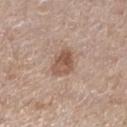Q: Was this lesion biopsied?
A: imaged on a skin check; not biopsied
Q: What kind of image is this?
A: ~15 mm crop, total-body skin-cancer survey
Q: Where on the body is the lesion?
A: the left lower leg
Q: Who is the patient?
A: female, aged 58 to 62
Q: What lighting was used for the tile?
A: white-light